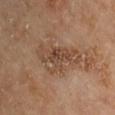follow-up: no biopsy performed (imaged during a skin exam); acquisition: ~15 mm crop, total-body skin-cancer survey; patient: male, in their mid- to late 60s; body site: the arm.